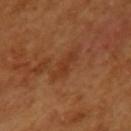Part of a total-body skin-imaging series; this lesion was reviewed on a skin check and was not flagged for biopsy.
The lesion is located on the left upper arm.
Measured at roughly 3.5 mm in maximum diameter.
Cropped from a whole-body photographic skin survey; the tile spans about 15 mm.
This is a cross-polarized tile.
A female patient, in their mid- to late 50s.